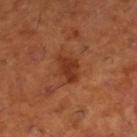Impression:
Captured during whole-body skin photography for melanoma surveillance; the lesion was not biopsied.
Image and clinical context:
Imaged with cross-polarized lighting. On the leg. The patient is a male aged around 65. A 15 mm crop from a total-body photograph taken for skin-cancer surveillance.